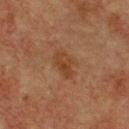The lesion was tiled from a total-body skin photograph and was not biopsied. The subject is a male about 75 years old. On the chest. Cropped from a total-body skin-imaging series; the visible field is about 15 mm. Imaged with cross-polarized lighting.